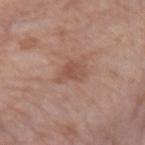{"biopsy_status": "not biopsied; imaged during a skin examination", "lighting": "white-light", "site": "left forearm", "patient": {"sex": "female", "age_approx": 50}, "lesion_size": {"long_diameter_mm_approx": 3.0}, "image": {"source": "total-body photography crop", "field_of_view_mm": 15}, "automated_metrics": {"border_irregularity_0_10": 4.0, "color_variation_0_10": 2.5, "peripheral_color_asymmetry": 0.5}}